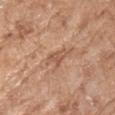<lesion>
  <biopsy_status>not biopsied; imaged during a skin examination</biopsy_status>
  <lighting>white-light</lighting>
  <site>right forearm</site>
  <patient>
    <sex>female</sex>
    <age_approx>75</age_approx>
  </patient>
  <lesion_size>
    <long_diameter_mm_approx>3.0</long_diameter_mm_approx>
  </lesion_size>
  <image>
    <source>total-body photography crop</source>
    <field_of_view_mm>15</field_of_view_mm>
  </image>
</lesion>